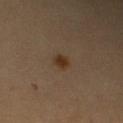Assessment:
Captured during whole-body skin photography for melanoma surveillance; the lesion was not biopsied.
Background:
Approximately 2 mm at its widest. The patient is a male roughly 60 years of age. Automated image analysis of the tile measured radial color variation of about 1. The lesion is located on the right upper arm. This image is a 15 mm lesion crop taken from a total-body photograph.